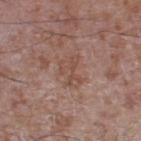{
  "patient": {
    "sex": "male",
    "age_approx": 60
  },
  "image": {
    "source": "total-body photography crop",
    "field_of_view_mm": 15
  },
  "automated_metrics": {
    "cielab_L": 49,
    "cielab_a": 19,
    "cielab_b": 25,
    "vs_skin_darker_L": 7.0,
    "vs_skin_contrast_norm": 5.5,
    "border_irregularity_0_10": 7.0,
    "peripheral_color_asymmetry": 0.5,
    "nevus_likeness_0_100": 0,
    "lesion_detection_confidence_0_100": 100
  },
  "lesion_size": {
    "long_diameter_mm_approx": 3.5
  },
  "site": "leg",
  "lighting": "white-light"
}